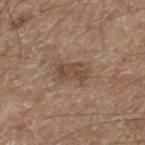Captured during whole-body skin photography for melanoma surveillance; the lesion was not biopsied. Approximately 3.5 mm at its widest. A male subject in their mid-60s. A lesion tile, about 15 mm wide, cut from a 3D total-body photograph. From the leg. The lesion-visualizer software estimated border irregularity of about 4 on a 0–10 scale, internal color variation of about 2.5 on a 0–10 scale, and a peripheral color-asymmetry measure near 1. The software also gave a nevus-likeness score of about 30/100 and a detector confidence of about 100 out of 100 that the crop contains a lesion.